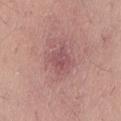biopsy_status: not biopsied; imaged during a skin examination
lesion_size:
  long_diameter_mm_approx: 2.5
patient:
  sex: male
  age_approx: 45
automated_metrics:
  area_mm2_approx: 5.5
  eccentricity: 0.55
  shape_asymmetry: 0.2
  cielab_L: 51
  cielab_a: 25
  cielab_b: 19
  vs_skin_darker_L: 8.0
  nevus_likeness_0_100: 0
  lesion_detection_confidence_0_100: 100
image:
  source: total-body photography crop
  field_of_view_mm: 15
site: left thigh
lighting: white-light